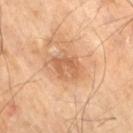Clinical impression:
Captured during whole-body skin photography for melanoma surveillance; the lesion was not biopsied.
Clinical summary:
A 15 mm close-up extracted from a 3D total-body photography capture. Automated image analysis of the tile measured a footprint of about 7 mm², an eccentricity of roughly 0.65, and a symmetry-axis asymmetry near 0.4. And it measured a classifier nevus-likeness of about 0/100 and a detector confidence of about 100 out of 100 that the crop contains a lesion. A male patient approximately 70 years of age. The lesion is located on the leg. The tile uses cross-polarized illumination.